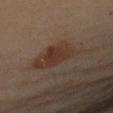Acquisition and patient details: A female subject about 60 years old. This is a cross-polarized tile. A 15 mm crop from a total-body photograph taken for skin-cancer surveillance. The lesion's longest dimension is about 7.5 mm. The lesion is on the left upper arm.